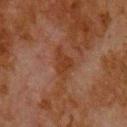Q: Was a biopsy performed?
A: no biopsy performed (imaged during a skin exam)
Q: Who is the patient?
A: male, aged 78–82
Q: Where on the body is the lesion?
A: the upper back
Q: Illumination type?
A: cross-polarized illumination
Q: What is the lesion's diameter?
A: ≈4 mm
Q: How was this image acquired?
A: ~15 mm tile from a whole-body skin photo